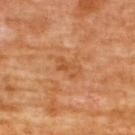Context:
A 15 mm crop from a total-body photograph taken for skin-cancer surveillance. The lesion is on the back. The total-body-photography lesion software estimated a border-irregularity index near 3.5/10, a color-variation rating of about 3/10, and radial color variation of about 1. The subject is a female aged around 65.